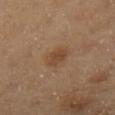<lesion>
<patient>
  <sex>female</sex>
  <age_approx>60</age_approx>
</patient>
<site>left lower leg</site>
<lesion_size>
  <long_diameter_mm_approx>3.0</long_diameter_mm_approx>
</lesion_size>
<automated_metrics>
  <area_mm2_approx>5.0</area_mm2_approx>
  <eccentricity>0.65</eccentricity>
  <shape_asymmetry>0.2</shape_asymmetry>
  <cielab_L>45</cielab_L>
  <cielab_a>19</cielab_a>
  <cielab_b>32</cielab_b>
  <vs_skin_darker_L>7.0</vs_skin_darker_L>
  <vs_skin_contrast_norm>6.0</vs_skin_contrast_norm>
  <border_irregularity_0_10>1.5</border_irregularity_0_10>
  <color_variation_0_10>2.0</color_variation_0_10>
  <peripheral_color_asymmetry>0.5</peripheral_color_asymmetry>
  <nevus_likeness_0_100>65</nevus_likeness_0_100>
</automated_metrics>
<image>
  <source>total-body photography crop</source>
  <field_of_view_mm>15</field_of_view_mm>
</image>
<lighting>cross-polarized</lighting>
</lesion>